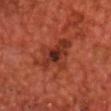Q: What is the anatomic site?
A: the chest
Q: Patient demographics?
A: male, approximately 70 years of age
Q: What is the imaging modality?
A: ~15 mm tile from a whole-body skin photo